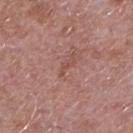Recorded during total-body skin imaging; not selected for excision or biopsy. A lesion tile, about 15 mm wide, cut from a 3D total-body photograph. On the upper back. The patient is a male roughly 65 years of age. Longest diameter approximately 2.5 mm. This is a white-light tile.